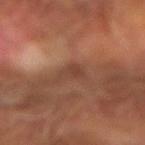diameter: ~2.5 mm (longest diameter) | acquisition: total-body-photography crop, ~15 mm field of view | image-analysis metrics: an average lesion color of about L≈39 a*≈20 b*≈27 (CIELAB), a lesion–skin lightness drop of about 7, and a lesion-to-skin contrast of about 6.5 (normalized; higher = more distinct); a border-irregularity rating of about 5/10, internal color variation of about 1 on a 0–10 scale, and peripheral color asymmetry of about 0.5 | anatomic site: the arm | lighting: cross-polarized illumination | subject: male, aged approximately 65.